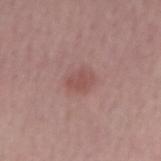Notes:
• imaging modality · ~15 mm crop, total-body skin-cancer survey
• patient · male, approximately 60 years of age
• size · ~3 mm (longest diameter)
• site · the right forearm
• illumination · white-light